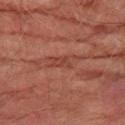follow-up: imaged on a skin check; not biopsied
patient: male, about 75 years old
image source: total-body-photography crop, ~15 mm field of view
anatomic site: the right thigh